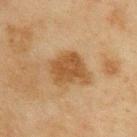Part of a total-body skin-imaging series; this lesion was reviewed on a skin check and was not flagged for biopsy.
About 4 mm across.
A male patient, approximately 45 years of age.
Located on the upper back.
A 15 mm crop from a total-body photograph taken for skin-cancer surveillance.
Captured under cross-polarized illumination.
The total-body-photography lesion software estimated a lesion area of about 10 mm², an outline eccentricity of about 0.65 (0 = round, 1 = elongated), and a shape-asymmetry score of about 0.25 (0 = symmetric). And it measured a lesion color around L≈41 a*≈17 b*≈33 in CIELAB. The software also gave a nevus-likeness score of about 95/100 and a detector confidence of about 100 out of 100 that the crop contains a lesion.